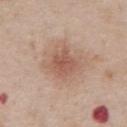| key | value |
|---|---|
| notes | catalogued during a skin exam; not biopsied |
| diameter | ~3 mm (longest diameter) |
| body site | the abdomen |
| subject | male, roughly 60 years of age |
| image source | ~15 mm tile from a whole-body skin photo |